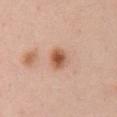Recorded during total-body skin imaging; not selected for excision or biopsy. The lesion's longest dimension is about 3 mm. An algorithmic analysis of the crop reported an area of roughly 5 mm² and a shape eccentricity near 0.55. The software also gave a classifier nevus-likeness of about 100/100 and a detector confidence of about 100 out of 100 that the crop contains a lesion. A 15 mm close-up tile from a total-body photography series done for melanoma screening. On the front of the torso. The subject is a female aged 48 to 52. Captured under white-light illumination.